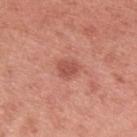Assessment:
Captured during whole-body skin photography for melanoma surveillance; the lesion was not biopsied.
Acquisition and patient details:
Automated image analysis of the tile measured a classifier nevus-likeness of about 70/100. Longest diameter approximately 2.5 mm. The tile uses white-light illumination. A roughly 15 mm field-of-view crop from a total-body skin photograph. A female patient aged 48 to 52. From the left upper arm.